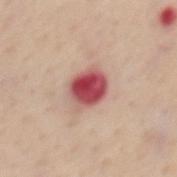biopsy_status: not biopsied; imaged during a skin examination
image:
  source: total-body photography crop
  field_of_view_mm: 15
site: mid back
automated_metrics:
  area_mm2_approx: 10.0
  eccentricity: 0.55
  cielab_L: 52
  cielab_a: 33
  cielab_b: 23
  vs_skin_darker_L: 19.0
  vs_skin_contrast_norm: 12.5
  border_irregularity_0_10: 2.0
  color_variation_0_10: 8.5
  peripheral_color_asymmetry: 3.0
patient:
  sex: female
  age_approx: 50
lesion_size:
  long_diameter_mm_approx: 4.0
lighting: white-light